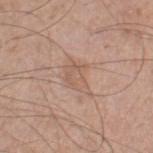{"biopsy_status": "not biopsied; imaged during a skin examination", "patient": {"sex": "male", "age_approx": 60}, "automated_metrics": {"vs_skin_darker_L": 6.0, "vs_skin_contrast_norm": 4.5, "color_variation_0_10": 2.5, "peripheral_color_asymmetry": 1.0, "nevus_likeness_0_100": 0, "lesion_detection_confidence_0_100": 100}, "lighting": "white-light", "lesion_size": {"long_diameter_mm_approx": 4.0}, "site": "right thigh", "image": {"source": "total-body photography crop", "field_of_view_mm": 15}}